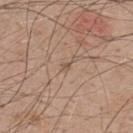tile lighting: white-light illumination
acquisition: total-body-photography crop, ~15 mm field of view
lesion diameter: ≈1 mm
image-analysis metrics: an eccentricity of roughly 0.55 and a shape-asymmetry score of about 0.5 (0 = symmetric); a lesion color around L≈53 a*≈16 b*≈29 in CIELAB and a lesion–skin lightness drop of about 8
subject: male, aged 48 to 52
site: the upper back